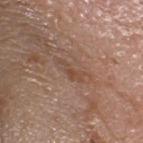Imaged during a routine full-body skin examination; the lesion was not biopsied and no histopathology is available.
Automated image analysis of the tile measured a border-irregularity rating of about 5/10, a color-variation rating of about 0/10, and peripheral color asymmetry of about 0. The software also gave an automated nevus-likeness rating near 0 out of 100 and lesion-presence confidence of about 95/100.
A female patient aged 68 to 72.
A close-up tile cropped from a whole-body skin photograph, about 15 mm across.
The lesion's longest dimension is about 3.5 mm.
On the head or neck.
Imaged with white-light lighting.